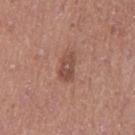Captured during whole-body skin photography for melanoma surveillance; the lesion was not biopsied. The subject is a female approximately 40 years of age. About 3.5 mm across. Captured under white-light illumination. The lesion is on the left thigh. A lesion tile, about 15 mm wide, cut from a 3D total-body photograph.